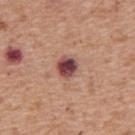  lighting: white-light
  image:
    source: total-body photography crop
    field_of_view_mm: 15
  site: upper back
  lesion_size:
    long_diameter_mm_approx: 2.5
  patient:
    sex: male
    age_approx: 70